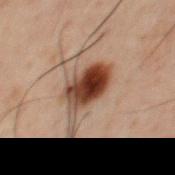Captured during whole-body skin photography for melanoma surveillance; the lesion was not biopsied.
Imaged with cross-polarized lighting.
About 5.5 mm across.
A male patient, about 60 years old.
The lesion is on the front of the torso.
Automated tile analysis of the lesion measured a lesion area of about 13 mm², an eccentricity of roughly 0.75, and two-axis asymmetry of about 0.3. It also reported a lesion color around L≈31 a*≈17 b*≈23 in CIELAB and a lesion-to-skin contrast of about 14 (normalized; higher = more distinct).
Cropped from a whole-body photographic skin survey; the tile spans about 15 mm.